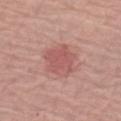Recorded during total-body skin imaging; not selected for excision or biopsy.
Located on the arm.
Cropped from a whole-body photographic skin survey; the tile spans about 15 mm.
The subject is a male roughly 70 years of age.
The tile uses white-light illumination.
Measured at roughly 4 mm in maximum diameter.